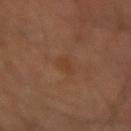Case summary:
– workup: imaged on a skin check; not biopsied
– patient: male, aged approximately 65
– body site: the left arm
– image-analysis metrics: a footprint of about 3.5 mm², an eccentricity of roughly 0.65, and a symmetry-axis asymmetry near 0.35; an average lesion color of about L≈37 a*≈20 b*≈29 (CIELAB), about 4 CIELAB-L* units darker than the surrounding skin, and a normalized lesion–skin contrast near 4.5
– image source: ~15 mm tile from a whole-body skin photo
– illumination: cross-polarized